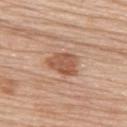notes: catalogued during a skin exam; not biopsied
illumination: white-light
image-analysis metrics: border irregularity of about 3 on a 0–10 scale, a within-lesion color-variation index near 2/10, and a peripheral color-asymmetry measure near 1
anatomic site: the back
image source: ~15 mm tile from a whole-body skin photo
subject: female, roughly 65 years of age
lesion size: ~4 mm (longest diameter)